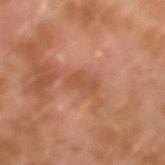{
  "biopsy_status": "not biopsied; imaged during a skin examination",
  "patient": {
    "sex": "male",
    "age_approx": 30
  },
  "site": "left forearm",
  "image": {
    "source": "total-body photography crop",
    "field_of_view_mm": 15
  }
}